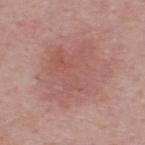Part of a total-body skin-imaging series; this lesion was reviewed on a skin check and was not flagged for biopsy. A 15 mm crop from a total-body photograph taken for skin-cancer surveillance. A male subject, roughly 50 years of age. On the back.